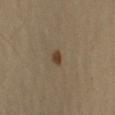Findings:
– tile lighting · cross-polarized
– TBP lesion metrics · a border-irregularity index near 3/10; a detector confidence of about 100 out of 100 that the crop contains a lesion
– size · ≈2 mm
– body site · the right upper arm
– subject · male, aged approximately 60
– image · ~15 mm tile from a whole-body skin photo